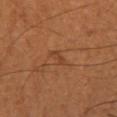{
  "biopsy_status": "not biopsied; imaged during a skin examination",
  "patient": {
    "sex": "female",
    "age_approx": 50
  },
  "automated_metrics": {
    "peripheral_color_asymmetry": 0.0,
    "nevus_likeness_0_100": 0
  },
  "site": "right upper arm",
  "image": {
    "source": "total-body photography crop",
    "field_of_view_mm": 15
  }
}